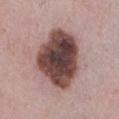Context:
Automated tile analysis of the lesion measured an area of roughly 32 mm² and an outline eccentricity of about 0.65 (0 = round, 1 = elongated). It also reported an average lesion color of about L≈43 a*≈19 b*≈20 (CIELAB) and a normalized border contrast of about 15. About 7.5 mm across. On the chest. A close-up tile cropped from a whole-body skin photograph, about 15 mm across. Captured under white-light illumination. The subject is a female aged 43–47.